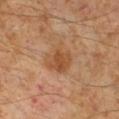Findings:
- notes · no biopsy performed (imaged during a skin exam)
- subject · male, aged 58 to 62
- body site · the leg
- image · ~15 mm tile from a whole-body skin photo
- illumination · cross-polarized illumination